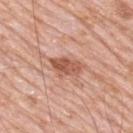Assessment: Recorded during total-body skin imaging; not selected for excision or biopsy. Acquisition and patient details: Automated image analysis of the tile measured an area of roughly 7 mm² and an eccentricity of roughly 0.85. The software also gave a border-irregularity index near 3/10 and radial color variation of about 1.5. The software also gave a nevus-likeness score of about 25/100 and a detector confidence of about 100 out of 100 that the crop contains a lesion. The subject is a male about 80 years old. Cropped from a total-body skin-imaging series; the visible field is about 15 mm. From the upper back. Imaged with white-light lighting.